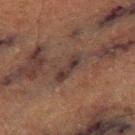Findings:
• body site · the right thigh
• patient · male, roughly 75 years of age
• automated lesion analysis · a nevus-likeness score of about 0/100 and a detector confidence of about 65 out of 100 that the crop contains a lesion
• lighting · cross-polarized illumination
• lesion diameter · about 4 mm
• acquisition · 15 mm crop, total-body photography